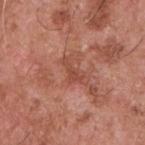biopsy status — catalogued during a skin exam; not biopsied